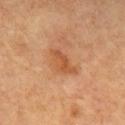Q: Was a biopsy performed?
A: catalogued during a skin exam; not biopsied
Q: Patient demographics?
A: female, approximately 60 years of age
Q: What kind of image is this?
A: 15 mm crop, total-body photography
Q: Where on the body is the lesion?
A: the chest
Q: Illumination type?
A: cross-polarized illumination